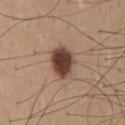Clinical impression:
No biopsy was performed on this lesion — it was imaged during a full skin examination and was not determined to be concerning.
Context:
Longest diameter approximately 4 mm. Cropped from a total-body skin-imaging series; the visible field is about 15 mm. A male subject, aged around 35. The tile uses white-light illumination. Located on the mid back.